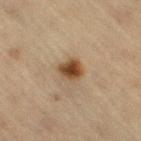anatomic site = the leg
lesion size = about 3 mm
subject = female, roughly 65 years of age
illumination = cross-polarized illumination
image source = ~15 mm tile from a whole-body skin photo